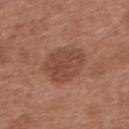| key | value |
|---|---|
| follow-up | imaged on a skin check; not biopsied |
| body site | the upper back |
| imaging modality | 15 mm crop, total-body photography |
| image-analysis metrics | a lesion area of about 17 mm²; a mean CIELAB color near L≈46 a*≈23 b*≈29, roughly 9 lightness units darker than nearby skin, and a normalized lesion–skin contrast near 6.5; a border-irregularity rating of about 2/10, internal color variation of about 3 on a 0–10 scale, and radial color variation of about 1; a classifier nevus-likeness of about 10/100 and a lesion-detection confidence of about 100/100 |
| subject | male, aged around 30 |
| illumination | white-light |